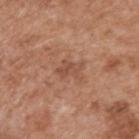No biopsy was performed on this lesion — it was imaged during a full skin examination and was not determined to be concerning. From the back. A 15 mm close-up extracted from a 3D total-body photography capture. This is a white-light tile. The subject is a male aged 63 to 67.